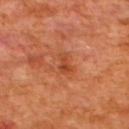| key | value |
|---|---|
| follow-up | total-body-photography surveillance lesion; no biopsy |
| acquisition | 15 mm crop, total-body photography |
| lesion diameter | ≈3 mm |
| location | the upper back |
| patient | male, in their mid- to late 60s |